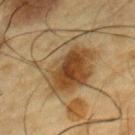Captured during whole-body skin photography for melanoma surveillance; the lesion was not biopsied. A male patient aged approximately 85 or older. Longest diameter approximately 6.5 mm. Automated image analysis of the tile measured an area of roughly 30 mm², an outline eccentricity of about 0.35 (0 = round, 1 = elongated), and two-axis asymmetry of about 0.25. The software also gave an automated nevus-likeness rating near 100 out of 100 and a lesion-detection confidence of about 100/100. From the chest. A 15 mm crop from a total-body photograph taken for skin-cancer surveillance. Captured under cross-polarized illumination.